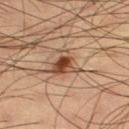| feature | finding |
|---|---|
| biopsy status | imaged on a skin check; not biopsied |
| patient | male, about 60 years old |
| image source | total-body-photography crop, ~15 mm field of view |
| site | the left thigh |
| tile lighting | cross-polarized illumination |